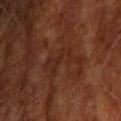Imaged during a routine full-body skin examination; the lesion was not biopsied and no histopathology is available.
The subject is a male roughly 65 years of age.
A close-up tile cropped from a whole-body skin photograph, about 15 mm across.
The lesion is located on the right upper arm.